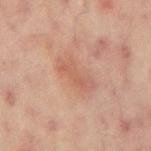The lesion was photographed on a routine skin check and not biopsied; there is no pathology result.
A 15 mm close-up extracted from a 3D total-body photography capture.
This is a cross-polarized tile.
The lesion is on the back.
Automated image analysis of the tile measured a lesion color around L≈54 a*≈22 b*≈28 in CIELAB, about 6 CIELAB-L* units darker than the surrounding skin, and a lesion-to-skin contrast of about 5 (normalized; higher = more distinct). It also reported a border-irregularity index near 4/10, a color-variation rating of about 3/10, and a peripheral color-asymmetry measure near 1. The analysis additionally found a detector confidence of about 100 out of 100 that the crop contains a lesion.
The lesion's longest dimension is about 4.5 mm.
The subject is a male in their 60s.